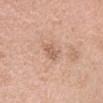Findings:
• automated metrics: a normalized border contrast of about 5; an automated nevus-likeness rating near 0 out of 100
• acquisition: 15 mm crop, total-body photography
• patient: female, aged approximately 35
• lesion diameter: ≈3 mm
• site: the head or neck
• lighting: white-light illumination